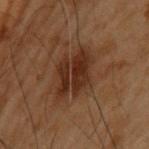Impression:
Imaged during a routine full-body skin examination; the lesion was not biopsied and no histopathology is available.
Acquisition and patient details:
The tile uses cross-polarized illumination. On the upper back. The patient is a male roughly 55 years of age. A 15 mm close-up extracted from a 3D total-body photography capture. The total-body-photography lesion software estimated a lesion area of about 14 mm², an outline eccentricity of about 0.7 (0 = round, 1 = elongated), and two-axis asymmetry of about 0.25. The analysis additionally found a detector confidence of about 100 out of 100 that the crop contains a lesion. About 5.5 mm across.